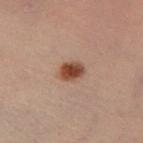Captured during whole-body skin photography for melanoma surveillance; the lesion was not biopsied.
A region of skin cropped from a whole-body photographic capture, roughly 15 mm wide.
A male subject, about 55 years old.
Automated image analysis of the tile measured an area of roughly 5.5 mm², a shape eccentricity near 0.7, and two-axis asymmetry of about 0.2. It also reported radial color variation of about 1.
The lesion is located on the left lower leg.